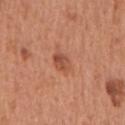Assessment:
The lesion was photographed on a routine skin check and not biopsied; there is no pathology result.
Acquisition and patient details:
Measured at roughly 3 mm in maximum diameter. A lesion tile, about 15 mm wide, cut from a 3D total-body photograph. The lesion-visualizer software estimated two-axis asymmetry of about 0.15. It also reported an average lesion color of about L≈51 a*≈27 b*≈33 (CIELAB), about 9 CIELAB-L* units darker than the surrounding skin, and a lesion-to-skin contrast of about 6.5 (normalized; higher = more distinct). It also reported a nevus-likeness score of about 85/100. The lesion is on the mid back. The tile uses white-light illumination. A male subject, aged around 65.